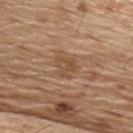notes = imaged on a skin check; not biopsied | image = ~15 mm crop, total-body skin-cancer survey | patient = male, about 70 years old | lesion size = about 3 mm | anatomic site = the upper back.